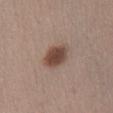Assessment:
Captured during whole-body skin photography for melanoma surveillance; the lesion was not biopsied.